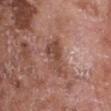<record>
<biopsy_status>not biopsied; imaged during a skin examination</biopsy_status>
<site>chest</site>
<patient>
  <sex>female</sex>
  <age_approx>75</age_approx>
</patient>
<image>
  <source>total-body photography crop</source>
  <field_of_view_mm>15</field_of_view_mm>
</image>
<lighting>white-light</lighting>
<lesion_size>
  <long_diameter_mm_approx>4.5</long_diameter_mm_approx>
</lesion_size>
</record>